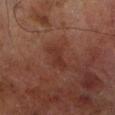Findings:
- workup — no biopsy performed (imaged during a skin exam)
- lesion diameter — about 3.5 mm
- image-analysis metrics — an area of roughly 4.5 mm², a shape eccentricity near 0.85, and two-axis asymmetry of about 0.3; a mean CIELAB color near L≈26 a*≈20 b*≈21, roughly 5 lightness units darker than nearby skin, and a normalized lesion–skin contrast near 5.5
- subject — male, in their 70s
- anatomic site — the left lower leg
- acquisition — total-body-photography crop, ~15 mm field of view
- lighting — cross-polarized